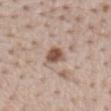workup = total-body-photography surveillance lesion; no biopsy
site = the mid back
subject = male, aged around 65
lighting = white-light illumination
automated lesion analysis = peripheral color asymmetry of about 1.5; a classifier nevus-likeness of about 95/100 and a lesion-detection confidence of about 100/100
acquisition = ~15 mm crop, total-body skin-cancer survey
diameter = about 2.5 mm